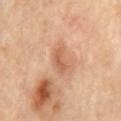Recorded during total-body skin imaging; not selected for excision or biopsy. From the chest. The patient is a female aged approximately 60. The total-body-photography lesion software estimated an average lesion color of about L≈60 a*≈23 b*≈34 (CIELAB), a lesion–skin lightness drop of about 10, and a normalized border contrast of about 6.5. The software also gave a border-irregularity rating of about 3.5/10, a within-lesion color-variation index near 2.5/10, and radial color variation of about 0.5. The analysis additionally found an automated nevus-likeness rating near 0 out of 100 and a detector confidence of about 100 out of 100 that the crop contains a lesion. Imaged with cross-polarized lighting. A 15 mm close-up extracted from a 3D total-body photography capture.